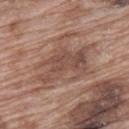Clinical impression: Captured during whole-body skin photography for melanoma surveillance; the lesion was not biopsied. Image and clinical context: This is a white-light tile. Located on the upper back. A roughly 15 mm field-of-view crop from a total-body skin photograph. A male subject in their 70s. Automated image analysis of the tile measured a footprint of about 16 mm². It also reported roughly 9 lightness units darker than nearby skin. The software also gave border irregularity of about 7 on a 0–10 scale and radial color variation of about 1.5. The software also gave a classifier nevus-likeness of about 0/100 and a detector confidence of about 60 out of 100 that the crop contains a lesion.